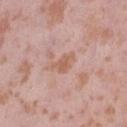The lesion was photographed on a routine skin check and not biopsied; there is no pathology result.
Longest diameter approximately 3 mm.
A female patient aged approximately 40.
Located on the right thigh.
A close-up tile cropped from a whole-body skin photograph, about 15 mm across.
Imaged with white-light lighting.